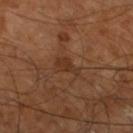patient:
  sex: male
  age_approx: 65
site: left lower leg
lesion_size:
  long_diameter_mm_approx: 3.0
automated_metrics:
  area_mm2_approx: 3.5
  eccentricity: 0.85
  shape_asymmetry: 0.45
  nevus_likeness_0_100: 0
  lesion_detection_confidence_0_100: 100
lighting: cross-polarized
image:
  source: total-body photography crop
  field_of_view_mm: 15